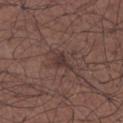Imaged during a routine full-body skin examination; the lesion was not biopsied and no histopathology is available. A male patient, aged 63 to 67. A lesion tile, about 15 mm wide, cut from a 3D total-body photograph. From the left forearm. This is a white-light tile.